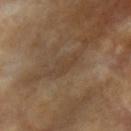The lesion was photographed on a routine skin check and not biopsied; there is no pathology result. The total-body-photography lesion software estimated a lesion area of about 4 mm² and an eccentricity of roughly 0.85. The software also gave a lesion color around L≈41 a*≈15 b*≈28 in CIELAB, roughly 5 lightness units darker than nearby skin, and a lesion-to-skin contrast of about 4.5 (normalized; higher = more distinct). The software also gave a border-irregularity rating of about 4.5/10 and a peripheral color-asymmetry measure near 0.5. Cropped from a total-body skin-imaging series; the visible field is about 15 mm. The subject is a female aged 73–77. Approximately 3 mm at its widest. The lesion is on the left upper arm.